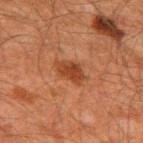Case summary:
- follow-up: no biopsy performed (imaged during a skin exam)
- patient: male, aged 58 to 62
- lesion size: ~3.5 mm (longest diameter)
- acquisition: ~15 mm crop, total-body skin-cancer survey
- anatomic site: the upper back
- illumination: cross-polarized